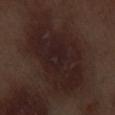{
  "biopsy_status": "not biopsied; imaged during a skin examination",
  "patient": {
    "sex": "male",
    "age_approx": 70
  },
  "automated_metrics": {
    "cielab_L": 20,
    "cielab_a": 15,
    "cielab_b": 15,
    "vs_skin_contrast_norm": 9.0,
    "border_irregularity_0_10": 3.0,
    "color_variation_0_10": 3.5,
    "peripheral_color_asymmetry": 1.0
  },
  "lesion_size": {
    "long_diameter_mm_approx": 9.5
  },
  "image": {
    "source": "total-body photography crop",
    "field_of_view_mm": 15
  },
  "site": "left thigh",
  "lighting": "white-light"
}